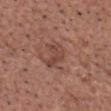Part of a total-body skin-imaging series; this lesion was reviewed on a skin check and was not flagged for biopsy. The patient is a male approximately 60 years of age. Approximately 3 mm at its widest. A region of skin cropped from a whole-body photographic capture, roughly 15 mm wide. The tile uses white-light illumination. On the head or neck.